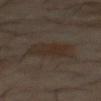Impression: This lesion was catalogued during total-body skin photography and was not selected for biopsy.